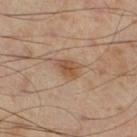Findings:
• follow-up — catalogued during a skin exam; not biopsied
• imaging modality — 15 mm crop, total-body photography
• image-analysis metrics — border irregularity of about 2.5 on a 0–10 scale and peripheral color asymmetry of about 1.5; a nevus-likeness score of about 55/100 and lesion-presence confidence of about 100/100
• lighting — cross-polarized
• location — the right lower leg
• lesion size — about 3 mm
• subject — male, in their mid-50s